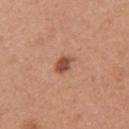Captured during whole-body skin photography for melanoma surveillance; the lesion was not biopsied.
Automated image analysis of the tile measured a shape eccentricity near 0.65 and two-axis asymmetry of about 0.3. And it measured a lesion color around L≈50 a*≈25 b*≈31 in CIELAB, a lesion–skin lightness drop of about 13, and a normalized border contrast of about 9. The software also gave a border-irregularity index near 2.5/10, a within-lesion color-variation index near 3/10, and radial color variation of about 1.
A female subject, in their mid-30s.
Cropped from a total-body skin-imaging series; the visible field is about 15 mm.
Located on the left upper arm.